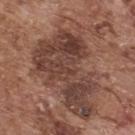Findings:
* subject · male, in their mid-70s
* illumination · white-light
* lesion diameter · about 12 mm
* imaging modality · ~15 mm tile from a whole-body skin photo
* location · the upper back